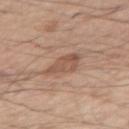{"biopsy_status": "not biopsied; imaged during a skin examination", "site": "right upper arm", "automated_metrics": {"area_mm2_approx": 6.5, "eccentricity": 0.75, "shape_asymmetry": 0.25, "border_irregularity_0_10": 2.5, "color_variation_0_10": 3.5, "nevus_likeness_0_100": 15, "lesion_detection_confidence_0_100": 100}, "image": {"source": "total-body photography crop", "field_of_view_mm": 15}, "patient": {"sex": "male", "age_approx": 45}}